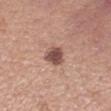  biopsy_status: not biopsied; imaged during a skin examination
  patient:
    sex: female
    age_approx: 35
  site: right forearm
  image:
    source: total-body photography crop
    field_of_view_mm: 15
  lesion_size:
    long_diameter_mm_approx: 3.0